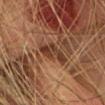<record>
<biopsy_status>not biopsied; imaged during a skin examination</biopsy_status>
<lighting>cross-polarized</lighting>
<site>head or neck</site>
<image>
  <source>total-body photography crop</source>
  <field_of_view_mm>15</field_of_view_mm>
</image>
<patient>
  <sex>female</sex>
  <age_approx>60</age_approx>
</patient>
<automated_metrics>
  <area_mm2_approx>7.0</area_mm2_approx>
  <eccentricity>0.85</eccentricity>
  <shape_asymmetry>0.4</shape_asymmetry>
  <vs_skin_darker_L>10.0</vs_skin_darker_L>
  <vs_skin_contrast_norm>9.0</vs_skin_contrast_norm>
  <color_variation_0_10>3.5</color_variation_0_10>
  <peripheral_color_asymmetry>1.0</peripheral_color_asymmetry>
  <nevus_likeness_0_100>0</nevus_likeness_0_100>
  <lesion_detection_confidence_0_100>55</lesion_detection_confidence_0_100>
</automated_metrics>
<lesion_size>
  <long_diameter_mm_approx>4.0</long_diameter_mm_approx>
</lesion_size>
</record>